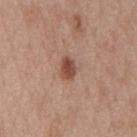Recorded during total-body skin imaging; not selected for excision or biopsy.
Captured under white-light illumination.
The subject is a female aged approximately 40.
From the front of the torso.
Cropped from a whole-body photographic skin survey; the tile spans about 15 mm.
The recorded lesion diameter is about 3 mm.